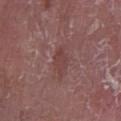Clinical impression: Recorded during total-body skin imaging; not selected for excision or biopsy. Clinical summary: A close-up tile cropped from a whole-body skin photograph, about 15 mm across. The lesion's longest dimension is about 3 mm. The lesion is located on the left lower leg. The subject is a male approximately 80 years of age. Captured under white-light illumination.